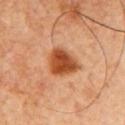image-analysis metrics: an outline eccentricity of about 0.5 (0 = round, 1 = elongated) and a shape-asymmetry score of about 0.3 (0 = symmetric); about 15 CIELAB-L* units darker than the surrounding skin and a normalized lesion–skin contrast near 11 | lesion size: ~4 mm (longest diameter) | subject: male, aged around 60 | lighting: cross-polarized illumination | imaging modality: total-body-photography crop, ~15 mm field of view | anatomic site: the chest.